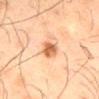workup: no biopsy performed (imaged during a skin exam)
lighting: cross-polarized illumination
image-analysis metrics: an eccentricity of roughly 0.75 and a shape-asymmetry score of about 0.25 (0 = symmetric); a mean CIELAB color near L≈62 a*≈25 b*≈39, roughly 15 lightness units darker than nearby skin, and a normalized border contrast of about 9; a border-irregularity index near 2.5/10 and a peripheral color-asymmetry measure near 1.5
image source: ~15 mm crop, total-body skin-cancer survey
site: the mid back
subject: male, aged approximately 45